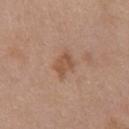No biopsy was performed on this lesion — it was imaged during a full skin examination and was not determined to be concerning.
From the chest.
A female patient, aged approximately 40.
A 15 mm close-up extracted from a 3D total-body photography capture.